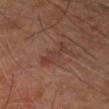This lesion was catalogued during total-body skin photography and was not selected for biopsy. This image is a 15 mm lesion crop taken from a total-body photograph. Automated image analysis of the tile measured a lesion area of about 5 mm², a shape eccentricity near 0.95, and a shape-asymmetry score of about 0.4 (0 = symmetric). The analysis additionally found an average lesion color of about L≈36 a*≈20 b*≈23 (CIELAB), about 6 CIELAB-L* units darker than the surrounding skin, and a normalized border contrast of about 5.5. It also reported a classifier nevus-likeness of about 10/100. The tile uses cross-polarized illumination. Measured at roughly 4 mm in maximum diameter. A male patient, aged 68 to 72. On the right forearm.